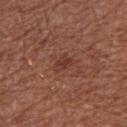No biopsy was performed on this lesion — it was imaged during a full skin examination and was not determined to be concerning.
The lesion is on the right upper arm.
Imaged with white-light lighting.
Automated tile analysis of the lesion measured a lesion area of about 4 mm² and an outline eccentricity of about 0.75 (0 = round, 1 = elongated). And it measured a mean CIELAB color near L≈38 a*≈23 b*≈26 and a normalized border contrast of about 5.5. It also reported a nevus-likeness score of about 0/100 and lesion-presence confidence of about 100/100.
A region of skin cropped from a whole-body photographic capture, roughly 15 mm wide.
About 3 mm across.
The patient is a male aged 63 to 67.